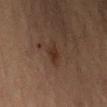Q: Is there a histopathology result?
A: imaged on a skin check; not biopsied
Q: Patient demographics?
A: male, aged around 55
Q: What kind of image is this?
A: ~15 mm tile from a whole-body skin photo
Q: What is the anatomic site?
A: the arm